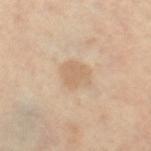The lesion was tiled from a total-body skin photograph and was not biopsied. The lesion is located on the right thigh. The subject is a female roughly 50 years of age. Cropped from a total-body skin-imaging series; the visible field is about 15 mm.